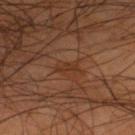location = the leg | lighting = cross-polarized | lesion diameter = about 2.5 mm | patient = male, aged approximately 55 | automated metrics = a border-irregularity rating of about 4/10, a within-lesion color-variation index near 0/10, and radial color variation of about 0; an automated nevus-likeness rating near 0 out of 100 and lesion-presence confidence of about 95/100 | image source = ~15 mm tile from a whole-body skin photo.